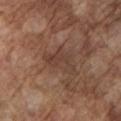Case summary:
– follow-up: imaged on a skin check; not biopsied
– subject: male, about 75 years old
– imaging modality: ~15 mm crop, total-body skin-cancer survey
– anatomic site: the left upper arm